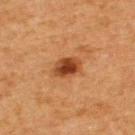Q: Was a biopsy performed?
A: no biopsy performed (imaged during a skin exam)
Q: What is the anatomic site?
A: the upper back
Q: Automated lesion metrics?
A: a lesion color around L≈38 a*≈24 b*≈35 in CIELAB, a lesion–skin lightness drop of about 13, and a normalized lesion–skin contrast near 10.5; a border-irregularity index near 1.5/10, a color-variation rating of about 5/10, and radial color variation of about 1
Q: What is the imaging modality?
A: total-body-photography crop, ~15 mm field of view
Q: What are the patient's age and sex?
A: male, aged approximately 65
Q: Illumination type?
A: cross-polarized illumination